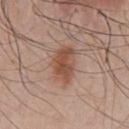Findings:
- illumination: white-light illumination
- patient: male, aged approximately 60
- site: the front of the torso
- TBP lesion metrics: an automated nevus-likeness rating near 95 out of 100
- imaging modality: ~15 mm tile from a whole-body skin photo